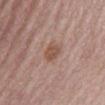A female patient, about 80 years old. The tile uses white-light illumination. The lesion-visualizer software estimated a lesion color around L≈52 a*≈20 b*≈26 in CIELAB, roughly 9 lightness units darker than nearby skin, and a normalized lesion–skin contrast near 7. It also reported a classifier nevus-likeness of about 25/100 and a lesion-detection confidence of about 100/100. A lesion tile, about 15 mm wide, cut from a 3D total-body photograph. Measured at roughly 3.5 mm in maximum diameter. From the mid back.